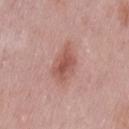site: the left thigh | automated lesion analysis: two-axis asymmetry of about 0.2; an average lesion color of about L≈54 a*≈25 b*≈25 (CIELAB) and roughly 10 lightness units darker than nearby skin; a classifier nevus-likeness of about 60/100 and lesion-presence confidence of about 100/100 | illumination: white-light | subject: female, about 40 years old | image: ~15 mm crop, total-body skin-cancer survey.